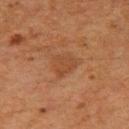Acquisition and patient details:
Located on the right thigh. This image is a 15 mm lesion crop taken from a total-body photograph. A female subject, in their 50s.